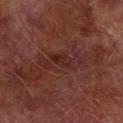Captured during whole-body skin photography for melanoma surveillance; the lesion was not biopsied. A region of skin cropped from a whole-body photographic capture, roughly 15 mm wide. The subject is a male in their mid-70s. Automated image analysis of the tile measured a within-lesion color-variation index near 3/10 and a peripheral color-asymmetry measure near 0.5. And it measured an automated nevus-likeness rating near 0 out of 100 and a lesion-detection confidence of about 95/100. The tile uses cross-polarized illumination. On the right forearm. Measured at roughly 5.5 mm in maximum diameter.